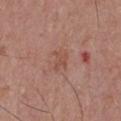biopsy status = total-body-photography surveillance lesion; no biopsy
lesion diameter = ~2.5 mm (longest diameter)
body site = the chest
illumination = white-light illumination
patient = male, in their 40s
acquisition = ~15 mm crop, total-body skin-cancer survey